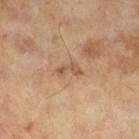{
  "biopsy_status": "not biopsied; imaged during a skin examination",
  "patient": {
    "sex": "male",
    "age_approx": 65
  },
  "image": {
    "source": "total-body photography crop",
    "field_of_view_mm": 15
  },
  "lesion_size": {
    "long_diameter_mm_approx": 3.0
  },
  "lighting": "cross-polarized"
}